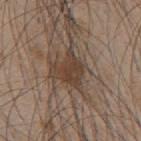Clinical impression:
The lesion was tiled from a total-body skin photograph and was not biopsied.
Acquisition and patient details:
The subject is a male aged around 45. Cropped from a total-body skin-imaging series; the visible field is about 15 mm. The lesion is located on the mid back.